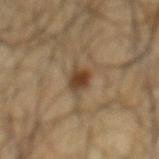Clinical summary:
The total-body-photography lesion software estimated a border-irregularity rating of about 3/10, a color-variation rating of about 3.5/10, and peripheral color asymmetry of about 1. Measured at roughly 3 mm in maximum diameter. A close-up tile cropped from a whole-body skin photograph, about 15 mm across. On the mid back. A male subject, aged approximately 65.